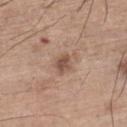Clinical impression:
Part of a total-body skin-imaging series; this lesion was reviewed on a skin check and was not flagged for biopsy.
Image and clinical context:
Automated tile analysis of the lesion measured a lesion area of about 4.5 mm², a shape eccentricity near 0.65, and a shape-asymmetry score of about 0.25 (0 = symmetric). The software also gave a border-irregularity index near 2.5/10, a color-variation rating of about 2.5/10, and radial color variation of about 1. The tile uses white-light illumination. A roughly 15 mm field-of-view crop from a total-body skin photograph. The lesion is located on the left lower leg. A male patient, aged around 65. Approximately 2.5 mm at its widest.